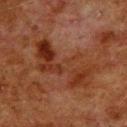Clinical impression:
This lesion was catalogued during total-body skin photography and was not selected for biopsy.
Image and clinical context:
A 15 mm crop from a total-body photograph taken for skin-cancer surveillance. Located on the upper back. Measured at roughly 9.5 mm in maximum diameter. The tile uses cross-polarized illumination. A male subject, aged approximately 80.